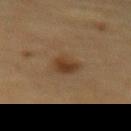<tbp_lesion>
<biopsy_status>not biopsied; imaged during a skin examination</biopsy_status>
<image>
  <source>total-body photography crop</source>
  <field_of_view_mm>15</field_of_view_mm>
</image>
<site>abdomen</site>
<lighting>cross-polarized</lighting>
<patient>
  <sex>male</sex>
  <age_approx>85+</age_approx>
</patient>
<lesion_size>
  <long_diameter_mm_approx>3.0</long_diameter_mm_approx>
</lesion_size>
</tbp_lesion>